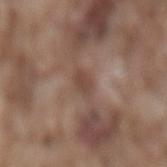{"lesion_size": {"long_diameter_mm_approx": 3.0}, "site": "back", "image": {"source": "total-body photography crop", "field_of_view_mm": 15}, "patient": {"sex": "male", "age_approx": 75}, "automated_metrics": {"border_irregularity_0_10": 3.0, "color_variation_0_10": 1.0, "peripheral_color_asymmetry": 0.5, "nevus_likeness_0_100": 0}}